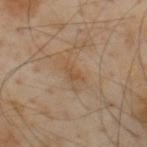This lesion was catalogued during total-body skin photography and was not selected for biopsy.
The lesion is on the upper back.
The total-body-photography lesion software estimated a footprint of about 2.5 mm² and a shape-asymmetry score of about 0.5 (0 = symmetric). The software also gave a normalized border contrast of about 5.5.
The tile uses cross-polarized illumination.
A lesion tile, about 15 mm wide, cut from a 3D total-body photograph.
A male patient in their mid- to late 50s.
The lesion's longest dimension is about 2.5 mm.